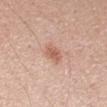Findings:
- workup — catalogued during a skin exam; not biopsied
- image source — 15 mm crop, total-body photography
- automated lesion analysis — a shape eccentricity near 0.6; a normalized border contrast of about 6.5; a nevus-likeness score of about 80/100 and lesion-presence confidence of about 100/100
- illumination — white-light
- location — the arm
- subject — female, aged around 25